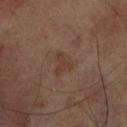notes = imaged on a skin check; not biopsied | TBP lesion metrics = a lesion color around L≈37 a*≈16 b*≈25 in CIELAB, about 5 CIELAB-L* units darker than the surrounding skin, and a normalized border contrast of about 5.5; lesion-presence confidence of about 100/100 | acquisition = ~15 mm crop, total-body skin-cancer survey | lighting = cross-polarized illumination | lesion size = ≈3 mm | body site = the right lower leg | subject = male, aged 68–72.